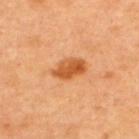The lesion was tiled from a total-body skin photograph and was not biopsied. Imaged with cross-polarized lighting. The lesion is located on the upper back. Approximately 4 mm at its widest. This image is a 15 mm lesion crop taken from a total-body photograph. A male patient, roughly 45 years of age.